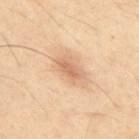Assessment: Imaged during a routine full-body skin examination; the lesion was not biopsied and no histopathology is available. Background: Captured under cross-polarized illumination. The lesion is located on the upper back. Longest diameter approximately 3.5 mm. The patient is a male aged approximately 55. Cropped from a whole-body photographic skin survey; the tile spans about 15 mm.